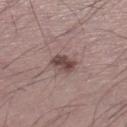The lesion was tiled from a total-body skin photograph and was not biopsied. Captured under white-light illumination. This image is a 15 mm lesion crop taken from a total-body photograph. The total-body-photography lesion software estimated a border-irregularity index near 2.5/10, a within-lesion color-variation index near 4/10, and a peripheral color-asymmetry measure near 1.5. The patient is a male aged 33–37. Approximately 3 mm at its widest. The lesion is on the left lower leg.